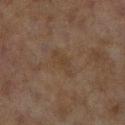Captured during whole-body skin photography for melanoma surveillance; the lesion was not biopsied. From the right lower leg. A roughly 15 mm field-of-view crop from a total-body skin photograph. The subject is a female in their 60s. Approximately 3.5 mm at its widest.